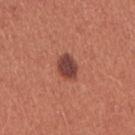Case summary:
* lighting · white-light
* body site · the arm
* diameter · ~3 mm (longest diameter)
* patient · female, aged 33–37
* imaging modality · total-body-photography crop, ~15 mm field of view